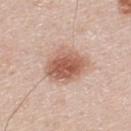This is a white-light tile. A male patient in their 40s. The lesion is located on the upper back. Cropped from a total-body skin-imaging series; the visible field is about 15 mm. Automated image analysis of the tile measured a border-irregularity rating of about 2/10, internal color variation of about 5 on a 0–10 scale, and peripheral color asymmetry of about 1.5.